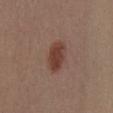Case summary:
- biopsy status: no biopsy performed (imaged during a skin exam)
- body site: the chest
- patient: female, aged around 30
- acquisition: ~15 mm tile from a whole-body skin photo